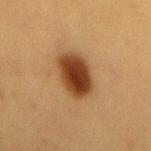| field | value |
|---|---|
| illumination | cross-polarized illumination |
| subject | female, aged 53 to 57 |
| size | ~5 mm (longest diameter) |
| automated metrics | an average lesion color of about L≈34 a*≈20 b*≈31 (CIELAB), about 15 CIELAB-L* units darker than the surrounding skin, and a normalized lesion–skin contrast near 13; a nevus-likeness score of about 100/100 and a detector confidence of about 100 out of 100 that the crop contains a lesion |
| image | ~15 mm crop, total-body skin-cancer survey |
| site | the mid back |